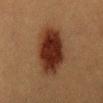- image · total-body-photography crop, ~15 mm field of view
- body site · the chest
- patient · female, about 40 years old